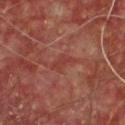A male subject in their mid- to late 60s.
The recorded lesion diameter is about 3 mm.
A close-up tile cropped from a whole-body skin photograph, about 15 mm across.
Automated image analysis of the tile measured border irregularity of about 5 on a 0–10 scale and internal color variation of about 1 on a 0–10 scale.
The tile uses cross-polarized illumination.
Located on the chest.